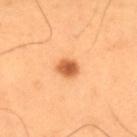biopsy status: total-body-photography surveillance lesion; no biopsy | subject: male, approximately 55 years of age | anatomic site: the mid back | acquisition: ~15 mm crop, total-body skin-cancer survey.